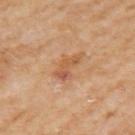workup: catalogued during a skin exam; not biopsied | acquisition: ~15 mm crop, total-body skin-cancer survey | size: about 4 mm | image-analysis metrics: a mean CIELAB color near L≈56 a*≈23 b*≈36 | site: the left upper arm | subject: female, in their 60s | tile lighting: cross-polarized.